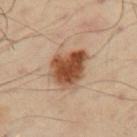Notes:
– follow-up: total-body-photography surveillance lesion; no biopsy
– imaging modality: ~15 mm tile from a whole-body skin photo
– lighting: cross-polarized
– TBP lesion metrics: a mean CIELAB color near L≈49 a*≈21 b*≈32 and a normalized border contrast of about 11.5; border irregularity of about 2.5 on a 0–10 scale and radial color variation of about 2
– lesion size: about 5 mm
– site: the left upper arm
– patient: male, roughly 50 years of age